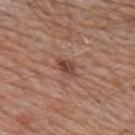Clinical impression: Part of a total-body skin-imaging series; this lesion was reviewed on a skin check and was not flagged for biopsy. Image and clinical context: From the back. This is a white-light tile. Cropped from a whole-body photographic skin survey; the tile spans about 15 mm. A male subject about 80 years old. Measured at roughly 2.5 mm in maximum diameter.